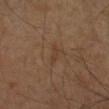No biopsy was performed on this lesion — it was imaged during a full skin examination and was not determined to be concerning.
A close-up tile cropped from a whole-body skin photograph, about 15 mm across.
The lesion is on the right lower leg.
Automated tile analysis of the lesion measured a footprint of about 2.5 mm², a shape eccentricity near 0.9, and two-axis asymmetry of about 0.4. The analysis additionally found an automated nevus-likeness rating near 0 out of 100 and lesion-presence confidence of about 100/100.
This is a cross-polarized tile.
The subject is a male roughly 60 years of age.